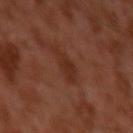Clinical impression:
The lesion was photographed on a routine skin check and not biopsied; there is no pathology result.